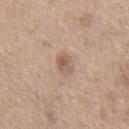<case>
<biopsy_status>not biopsied; imaged during a skin examination</biopsy_status>
<automated_metrics>
  <area_mm2_approx>4.0</area_mm2_approx>
  <eccentricity>0.7</eccentricity>
  <nevus_likeness_0_100>25</nevus_likeness_0_100>
  <lesion_detection_confidence_0_100>100</lesion_detection_confidence_0_100>
</automated_metrics>
<lighting>white-light</lighting>
<image>
  <source>total-body photography crop</source>
  <field_of_view_mm>15</field_of_view_mm>
</image>
<patient>
  <sex>male</sex>
  <age_approx>65</age_approx>
</patient>
<site>chest</site>
</case>